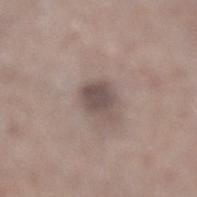{
  "biopsy_status": "not biopsied; imaged during a skin examination",
  "site": "left lower leg",
  "patient": {
    "sex": "male",
    "age_approx": 65
  },
  "image": {
    "source": "total-body photography crop",
    "field_of_view_mm": 15
  },
  "lesion_size": {
    "long_diameter_mm_approx": 3.0
  },
  "lighting": "white-light",
  "automated_metrics": {
    "eccentricity": 0.5,
    "shape_asymmetry": 0.2,
    "nevus_likeness_0_100": 10,
    "lesion_detection_confidence_0_100": 100
  }
}